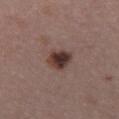Assessment: No biopsy was performed on this lesion — it was imaged during a full skin examination and was not determined to be concerning. Acquisition and patient details: The patient is a female aged around 30. A 15 mm crop from a total-body photograph taken for skin-cancer surveillance. Approximately 3 mm at its widest. On the upper back. Captured under white-light illumination.